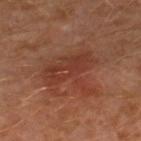Clinical impression:
Part of a total-body skin-imaging series; this lesion was reviewed on a skin check and was not flagged for biopsy.
Context:
A male subject, aged around 30. Located on the left leg. Captured under cross-polarized illumination. Cropped from a whole-body photographic skin survey; the tile spans about 15 mm.